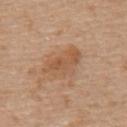Clinical impression: The lesion was photographed on a routine skin check and not biopsied; there is no pathology result. Background: The lesion-visualizer software estimated an eccentricity of roughly 0.85 and a shape-asymmetry score of about 0.3 (0 = symmetric). It also reported a lesion color around L≈55 a*≈20 b*≈34 in CIELAB, about 8 CIELAB-L* units darker than the surrounding skin, and a normalized border contrast of about 6. And it measured a border-irregularity index near 4/10 and a color-variation rating of about 3.5/10. The analysis additionally found a nevus-likeness score of about 35/100 and a lesion-detection confidence of about 100/100. A male subject approximately 70 years of age. A 15 mm close-up tile from a total-body photography series done for melanoma screening. About 5.5 mm across. Imaged with white-light lighting. From the mid back.